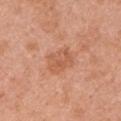Findings:
- follow-up · catalogued during a skin exam; not biopsied
- patient · female, in their 40s
- image · ~15 mm crop, total-body skin-cancer survey
- body site · the arm